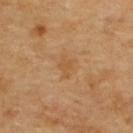Part of a total-body skin-imaging series; this lesion was reviewed on a skin check and was not flagged for biopsy.
Cropped from a total-body skin-imaging series; the visible field is about 15 mm.
Approximately 3 mm at its widest.
Automated image analysis of the tile measured an area of roughly 3.5 mm², a shape eccentricity near 0.8, and a shape-asymmetry score of about 0.25 (0 = symmetric). And it measured a nevus-likeness score of about 0/100.
The lesion is on the upper back.
The patient is aged approximately 60.
This is a cross-polarized tile.